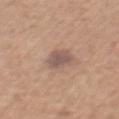A roughly 15 mm field-of-view crop from a total-body skin photograph. From the abdomen. The lesion's longest dimension is about 3 mm. Imaged with white-light lighting. The subject is a male in their mid- to late 50s.